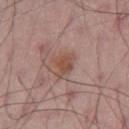Q: Was a biopsy performed?
A: catalogued during a skin exam; not biopsied
Q: Who is the patient?
A: male, aged around 55
Q: How was this image acquired?
A: ~15 mm crop, total-body skin-cancer survey
Q: Automated lesion metrics?
A: a footprint of about 6 mm², an eccentricity of roughly 0.45, and a shape-asymmetry score of about 0.35 (0 = symmetric); border irregularity of about 3.5 on a 0–10 scale, a color-variation rating of about 4/10, and peripheral color asymmetry of about 1.5
Q: Lesion location?
A: the right thigh